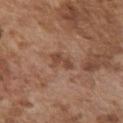Captured during whole-body skin photography for melanoma surveillance; the lesion was not biopsied. The patient is a male aged 73 to 77. On the chest. Cropped from a total-body skin-imaging series; the visible field is about 15 mm. Captured under white-light illumination.